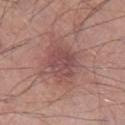Recorded during total-body skin imaging; not selected for excision or biopsy.
Longest diameter approximately 3.5 mm.
A region of skin cropped from a whole-body photographic capture, roughly 15 mm wide.
The lesion-visualizer software estimated a mean CIELAB color near L≈48 a*≈23 b*≈20, a lesion–skin lightness drop of about 7, and a normalized border contrast of about 6.
Located on the right lower leg.
A male patient about 70 years old.
Imaged with white-light lighting.